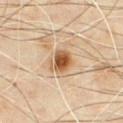Q: Was this lesion biopsied?
A: no biopsy performed (imaged during a skin exam)
Q: What kind of image is this?
A: ~15 mm tile from a whole-body skin photo
Q: What are the patient's age and sex?
A: male, aged 63 to 67
Q: Illumination type?
A: cross-polarized illumination
Q: What did automated image analysis measure?
A: a border-irregularity index near 3/10 and a color-variation rating of about 5.5/10; a nevus-likeness score of about 95/100 and lesion-presence confidence of about 100/100
Q: What is the lesion's diameter?
A: about 3.5 mm
Q: What is the anatomic site?
A: the chest